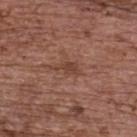lesion size = ~3 mm (longest diameter)
imaging modality = ~15 mm crop, total-body skin-cancer survey
subject = female, aged around 65
body site = the upper back